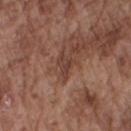follow-up — catalogued during a skin exam; not biopsied
image — ~15 mm crop, total-body skin-cancer survey
illumination — white-light illumination
subject — male, aged 73–77
site — the right upper arm